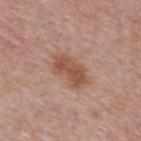Impression: Part of a total-body skin-imaging series; this lesion was reviewed on a skin check and was not flagged for biopsy. Clinical summary: The lesion is located on the back. The subject is a male in their mid- to late 50s. The total-body-photography lesion software estimated an outline eccentricity of about 0.85 (0 = round, 1 = elongated) and a symmetry-axis asymmetry near 0.25. It also reported a border-irregularity rating of about 3/10, internal color variation of about 3 on a 0–10 scale, and peripheral color asymmetry of about 1. It also reported a classifier nevus-likeness of about 45/100 and a lesion-detection confidence of about 100/100. A 15 mm close-up extracted from a 3D total-body photography capture. The tile uses white-light illumination.